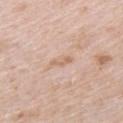{
  "biopsy_status": "not biopsied; imaged during a skin examination",
  "site": "left upper arm",
  "lesion_size": {
    "long_diameter_mm_approx": 3.0
  },
  "image": {
    "source": "total-body photography crop",
    "field_of_view_mm": 15
  },
  "patient": {
    "sex": "male",
    "age_approx": 50
  }
}